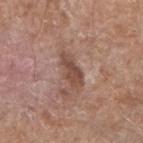Impression:
The lesion was photographed on a routine skin check and not biopsied; there is no pathology result.
Image and clinical context:
A male subject, about 60 years old. A 15 mm close-up extracted from a 3D total-body photography capture. Imaged with white-light lighting. Located on the arm. Measured at roughly 4.5 mm in maximum diameter. An algorithmic analysis of the crop reported a footprint of about 6.5 mm² and two-axis asymmetry of about 0.25.